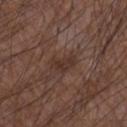Q: Was this lesion biopsied?
A: imaged on a skin check; not biopsied
Q: What did automated image analysis measure?
A: a nevus-likeness score of about 5/100 and a lesion-detection confidence of about 90/100
Q: What kind of image is this?
A: ~15 mm tile from a whole-body skin photo
Q: What is the anatomic site?
A: the left forearm
Q: Lesion size?
A: about 3 mm
Q: Who is the patient?
A: male, aged approximately 50
Q: How was the tile lit?
A: white-light illumination